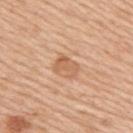The lesion was photographed on a routine skin check and not biopsied; there is no pathology result. A female patient roughly 45 years of age. A lesion tile, about 15 mm wide, cut from a 3D total-body photograph. The recorded lesion diameter is about 3 mm. Automated tile analysis of the lesion measured an area of roughly 5 mm², an outline eccentricity of about 0.65 (0 = round, 1 = elongated), and a shape-asymmetry score of about 0.35 (0 = symmetric). It also reported an automated nevus-likeness rating near 15 out of 100 and a lesion-detection confidence of about 100/100. On the right upper arm. Imaged with white-light lighting.